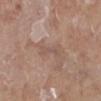<record>
<biopsy_status>not biopsied; imaged during a skin examination</biopsy_status>
<patient>
  <sex>female</sex>
  <age_approx>75</age_approx>
</patient>
<image>
  <source>total-body photography crop</source>
  <field_of_view_mm>15</field_of_view_mm>
</image>
<site>right lower leg</site>
<lesion_size>
  <long_diameter_mm_approx>3.5</long_diameter_mm_approx>
</lesion_size>
</record>